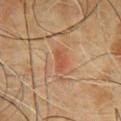The lesion was tiled from a total-body skin photograph and was not biopsied. The tile uses cross-polarized illumination. Cropped from a total-body skin-imaging series; the visible field is about 15 mm. The subject is a male in their 60s. From the front of the torso.